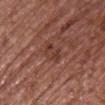Clinical impression:
Recorded during total-body skin imaging; not selected for excision or biopsy.
Image and clinical context:
A close-up tile cropped from a whole-body skin photograph, about 15 mm across. From the chest. A female subject, aged around 65.